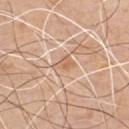<lesion>
<biopsy_status>not biopsied; imaged during a skin examination</biopsy_status>
<image>
  <source>total-body photography crop</source>
  <field_of_view_mm>15</field_of_view_mm>
</image>
<patient>
  <sex>male</sex>
  <age_approx>50</age_approx>
</patient>
<site>chest</site>
</lesion>